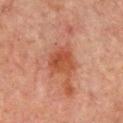Findings:
* workup — no biopsy performed (imaged during a skin exam)
* imaging modality — total-body-photography crop, ~15 mm field of view
* lesion diameter — ~4 mm (longest diameter)
* TBP lesion metrics — an area of roughly 11 mm², an outline eccentricity of about 0.45 (0 = round, 1 = elongated), and a symmetry-axis asymmetry near 0.3; border irregularity of about 3 on a 0–10 scale, internal color variation of about 4 on a 0–10 scale, and radial color variation of about 1.5; a classifier nevus-likeness of about 10/100
* anatomic site — the upper back
* subject — male, aged approximately 65
* tile lighting — cross-polarized